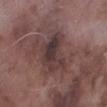TBP lesion metrics: an eccentricity of roughly 0.7 and a shape-asymmetry score of about 0.35 (0 = symmetric); a lesion–skin lightness drop of about 9 and a normalized border contrast of about 8.5; border irregularity of about 4 on a 0–10 scale and radial color variation of about 2 | acquisition: ~15 mm tile from a whole-body skin photo | subject: male, in their mid- to late 70s | lesion diameter: about 5 mm | location: the right lower leg.